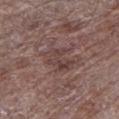site = the left lower leg
acquisition = ~15 mm crop, total-body skin-cancer survey
lighting = white-light
subject = male, aged around 70